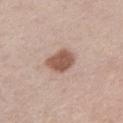{
  "biopsy_status": "not biopsied; imaged during a skin examination",
  "image": {
    "source": "total-body photography crop",
    "field_of_view_mm": 15
  },
  "lighting": "white-light",
  "patient": {
    "sex": "male",
    "age_approx": 60
  },
  "site": "left lower leg"
}